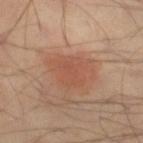Assessment:
Part of a total-body skin-imaging series; this lesion was reviewed on a skin check and was not flagged for biopsy.
Clinical summary:
The total-body-photography lesion software estimated a lesion area of about 9.5 mm² and an outline eccentricity of about 0.65 (0 = round, 1 = elongated). The analysis additionally found a nevus-likeness score of about 40/100 and a detector confidence of about 100 out of 100 that the crop contains a lesion. A male patient, aged 43 to 47. The lesion's longest dimension is about 3.5 mm. Imaged with cross-polarized lighting. A 15 mm crop from a total-body photograph taken for skin-cancer surveillance. On the right thigh.